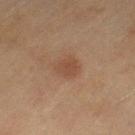Assessment: The lesion was photographed on a routine skin check and not biopsied; there is no pathology result. Image and clinical context: The total-body-photography lesion software estimated an area of roughly 4.5 mm², a shape eccentricity near 0.6, and a shape-asymmetry score of about 0.3 (0 = symmetric). The analysis additionally found an average lesion color of about L≈43 a*≈19 b*≈28 (CIELAB), roughly 7 lightness units darker than nearby skin, and a normalized lesion–skin contrast near 5.5. The software also gave a within-lesion color-variation index near 1.5/10 and a peripheral color-asymmetry measure near 0.5. The software also gave a classifier nevus-likeness of about 60/100 and a detector confidence of about 100 out of 100 that the crop contains a lesion. Longest diameter approximately 2.5 mm. A female subject, about 60 years old. The tile uses cross-polarized illumination. A region of skin cropped from a whole-body photographic capture, roughly 15 mm wide. Located on the left thigh.